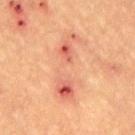Impression: Captured during whole-body skin photography for melanoma surveillance; the lesion was not biopsied. Context: Longest diameter approximately 8.5 mm. A male patient aged approximately 55. A 15 mm close-up tile from a total-body photography series done for melanoma screening. This is a cross-polarized tile. The lesion is on the mid back.